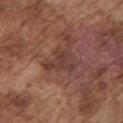workup: imaged on a skin check; not biopsied | automated lesion analysis: an area of roughly 8.5 mm², a shape eccentricity near 0.7, and a symmetry-axis asymmetry near 0.5; a border-irregularity index near 5/10, a color-variation rating of about 2.5/10, and radial color variation of about 0.5; a classifier nevus-likeness of about 0/100 and a lesion-detection confidence of about 100/100 | patient: male, roughly 75 years of age | anatomic site: the left upper arm | lighting: white-light | size: about 4.5 mm | image: 15 mm crop, total-body photography.